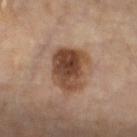Clinical summary:
Longest diameter approximately 5 mm. A 15 mm close-up extracted from a 3D total-body photography capture. A female subject aged approximately 75. On the right thigh. Imaged with cross-polarized lighting.